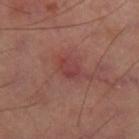Recorded during total-body skin imaging; not selected for excision or biopsy. A 15 mm close-up extracted from a 3D total-body photography capture. On the right thigh.